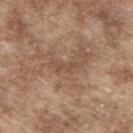Imaged during a routine full-body skin examination; the lesion was not biopsied and no histopathology is available. Approximately 3 mm at its widest. A male subject, about 65 years old. The lesion is on the upper back. A 15 mm close-up tile from a total-body photography series done for melanoma screening.